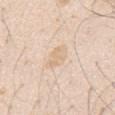Clinical summary:
Automated tile analysis of the lesion measured an area of roughly 6 mm² and two-axis asymmetry of about 0.25. A 15 mm close-up tile from a total-body photography series done for melanoma screening. The lesion is located on the chest. Imaged with white-light lighting. The patient is a male in their mid-40s.